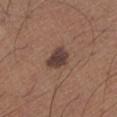{"biopsy_status": "not biopsied; imaged during a skin examination", "lesion_size": {"long_diameter_mm_approx": 3.0}, "automated_metrics": {"area_mm2_approx": 6.0, "eccentricity": 0.6, "cielab_L": 39, "cielab_a": 17, "cielab_b": 21, "vs_skin_contrast_norm": 11.0}, "image": {"source": "total-body photography crop", "field_of_view_mm": 15}, "lighting": "white-light", "site": "left lower leg", "patient": {"sex": "male", "age_approx": 65}}